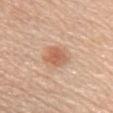Assessment:
The lesion was photographed on a routine skin check and not biopsied; there is no pathology result.
Background:
A 15 mm close-up extracted from a 3D total-body photography capture. This is a white-light tile. The recorded lesion diameter is about 3 mm. A female patient aged 63–67. The lesion is located on the chest.